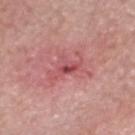An algorithmic analysis of the crop reported a lesion area of about 9.5 mm². It also reported an average lesion color of about L≈54 a*≈31 b*≈22 (CIELAB) and about 9 CIELAB-L* units darker than the surrounding skin. And it measured a classifier nevus-likeness of about 0/100 and lesion-presence confidence of about 100/100. The recorded lesion diameter is about 4.5 mm. The patient is a male about 80 years old. On the head or neck. A 15 mm close-up extracted from a 3D total-body photography capture.